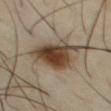  biopsy_status: not biopsied; imaged during a skin examination
  lesion_size:
    long_diameter_mm_approx: 6.5
  site: chest
  patient:
    sex: male
    age_approx: 55
  image:
    source: total-body photography crop
    field_of_view_mm: 15
  lighting: cross-polarized